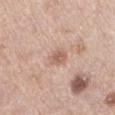Q: Was a biopsy performed?
A: no biopsy performed (imaged during a skin exam)
Q: Patient demographics?
A: female, roughly 65 years of age
Q: How was this image acquired?
A: ~15 mm crop, total-body skin-cancer survey
Q: How was the tile lit?
A: white-light
Q: Where on the body is the lesion?
A: the right thigh
Q: Automated lesion metrics?
A: a footprint of about 6 mm²; border irregularity of about 2.5 on a 0–10 scale, a color-variation rating of about 2.5/10, and radial color variation of about 0.5; a nevus-likeness score of about 0/100 and a detector confidence of about 100 out of 100 that the crop contains a lesion
Q: Lesion size?
A: about 3 mm